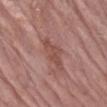A roughly 15 mm field-of-view crop from a total-body skin photograph. From the left forearm. This is a white-light tile. A female patient, aged 63 to 67. The recorded lesion diameter is about 4 mm.